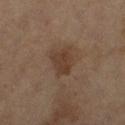Notes:
• subject — female, aged approximately 60
• site — the left thigh
• acquisition — ~15 mm tile from a whole-body skin photo